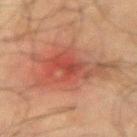follow-up: catalogued during a skin exam; not biopsied | diameter: ~8 mm (longest diameter) | subject: male, approximately 70 years of age | image: 15 mm crop, total-body photography | body site: the right forearm | lighting: cross-polarized.